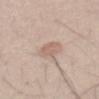No biopsy was performed on this lesion — it was imaged during a full skin examination and was not determined to be concerning. A male patient in their mid- to late 30s. Imaged with white-light lighting. An algorithmic analysis of the crop reported a border-irregularity rating of about 3/10, a color-variation rating of about 1.5/10, and peripheral color asymmetry of about 0.5. It also reported an automated nevus-likeness rating near 35 out of 100. Located on the front of the torso. Longest diameter approximately 3 mm. A 15 mm close-up extracted from a 3D total-body photography capture.